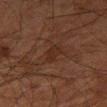Case summary:
– notes · total-body-photography surveillance lesion; no biopsy
– image source · ~15 mm crop, total-body skin-cancer survey
– anatomic site · the right thigh
– diameter · about 3 mm
– patient · male, aged approximately 80